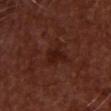follow-up: imaged on a skin check; not biopsied | size: about 3 mm | automated lesion analysis: a mean CIELAB color near L≈19 a*≈22 b*≈23, about 6 CIELAB-L* units darker than the surrounding skin, and a lesion-to-skin contrast of about 7 (normalized; higher = more distinct); border irregularity of about 3.5 on a 0–10 scale, a color-variation rating of about 1.5/10, and peripheral color asymmetry of about 0.5 | lighting: cross-polarized | site: the chest | acquisition: ~15 mm tile from a whole-body skin photo | patient: male, aged 48 to 52.